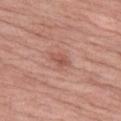Case summary:
– biopsy status: total-body-photography surveillance lesion; no biopsy